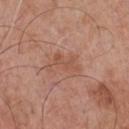<case>
  <image>
    <source>total-body photography crop</source>
    <field_of_view_mm>15</field_of_view_mm>
  </image>
  <site>chest</site>
  <lesion_size>
    <long_diameter_mm_approx>4.5</long_diameter_mm_approx>
  </lesion_size>
  <lighting>white-light</lighting>
  <patient>
    <sex>male</sex>
    <age_approx>70</age_approx>
  </patient>
</case>